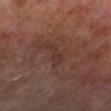Clinical impression:
Captured during whole-body skin photography for melanoma surveillance; the lesion was not biopsied.
Acquisition and patient details:
A close-up tile cropped from a whole-body skin photograph, about 15 mm across. Imaged with cross-polarized lighting. A male subject, approximately 70 years of age. From the leg. The lesion-visualizer software estimated a mean CIELAB color near L≈32 a*≈18 b*≈22, a lesion–skin lightness drop of about 5, and a normalized border contrast of about 5. It also reported an automated nevus-likeness rating near 0 out of 100 and a detector confidence of about 85 out of 100 that the crop contains a lesion.